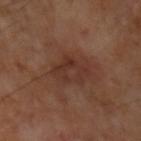Clinical summary: The lesion is on the left upper arm. Captured under cross-polarized illumination. Measured at roughly 4 mm in maximum diameter. A region of skin cropped from a whole-body photographic capture, roughly 15 mm wide.